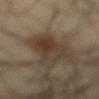This lesion was catalogued during total-body skin photography and was not selected for biopsy. The subject is a male about 40 years old. This is a cross-polarized tile. On the front of the torso. This image is a 15 mm lesion crop taken from a total-body photograph.